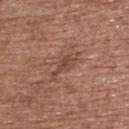Part of a total-body skin-imaging series; this lesion was reviewed on a skin check and was not flagged for biopsy. On the upper back. A female subject approximately 80 years of age. This is a white-light tile. A region of skin cropped from a whole-body photographic capture, roughly 15 mm wide.